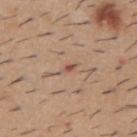biopsy_status: not biopsied; imaged during a skin examination
lesion_size:
  long_diameter_mm_approx: 2.5
patient:
  sex: male
  age_approx: 60
image:
  source: total-body photography crop
  field_of_view_mm: 15
site: upper back
automated_metrics:
  area_mm2_approx: 2.0
  eccentricity: 0.9
  shape_asymmetry: 0.45
  border_irregularity_0_10: 4.5
  color_variation_0_10: 0.0
lighting: white-light